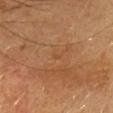The lesion was tiled from a total-body skin photograph and was not biopsied. A female subject, aged approximately 55. A close-up tile cropped from a whole-body skin photograph, about 15 mm across. On the head or neck. The tile uses cross-polarized illumination.